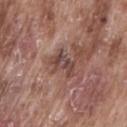biopsy_status: not biopsied; imaged during a skin examination
site: lower back
lesion_size:
  long_diameter_mm_approx: 3.5
patient:
  sex: male
  age_approx: 75
image:
  source: total-body photography crop
  field_of_view_mm: 15